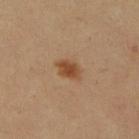follow-up — catalogued during a skin exam; not biopsied
subject — female, about 45 years old
automated lesion analysis — roughly 10 lightness units darker than nearby skin and a lesion-to-skin contrast of about 8.5 (normalized; higher = more distinct)
tile lighting — cross-polarized illumination
diameter — about 3 mm
site — the right lower leg
imaging modality — ~15 mm tile from a whole-body skin photo